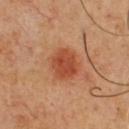<tbp_lesion>
  <biopsy_status>not biopsied; imaged during a skin examination</biopsy_status>
  <site>front of the torso</site>
  <automated_metrics>
    <area_mm2_approx>10.0</area_mm2_approx>
    <eccentricity>0.5</eccentricity>
    <nevus_likeness_0_100>100</nevus_likeness_0_100>
  </automated_metrics>
  <lighting>cross-polarized</lighting>
  <image>
    <source>total-body photography crop</source>
    <field_of_view_mm>15</field_of_view_mm>
  </image>
  <patient>
    <sex>male</sex>
    <age_approx>50</age_approx>
  </patient>
  <lesion_size>
    <long_diameter_mm_approx>4.0</long_diameter_mm_approx>
  </lesion_size>
</tbp_lesion>